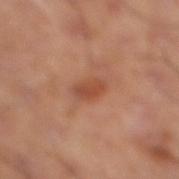| key | value |
|---|---|
| workup | no biopsy performed (imaged during a skin exam) |
| location | the leg |
| lighting | cross-polarized |
| diameter | about 3.5 mm |
| automated lesion analysis | a mean CIELAB color near L≈50 a*≈26 b*≈33 and a lesion-to-skin contrast of about 6.5 (normalized; higher = more distinct); a border-irregularity index near 1.5/10, a within-lesion color-variation index near 4/10, and peripheral color asymmetry of about 1.5; an automated nevus-likeness rating near 35 out of 100 and a detector confidence of about 100 out of 100 that the crop contains a lesion |
| acquisition | ~15 mm tile from a whole-body skin photo |